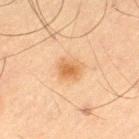notes = no biopsy performed (imaged during a skin exam)
image = total-body-photography crop, ~15 mm field of view
patient = male, aged 63 to 67
illumination = cross-polarized
TBP lesion metrics = a border-irregularity rating of about 1.5/10, a color-variation rating of about 3.5/10, and peripheral color asymmetry of about 1; a classifier nevus-likeness of about 95/100
size = about 3 mm
body site = the leg